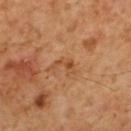Q: Was this lesion biopsied?
A: catalogued during a skin exam; not biopsied
Q: Patient demographics?
A: male, aged 58 to 62
Q: What is the anatomic site?
A: the mid back
Q: What kind of image is this?
A: 15 mm crop, total-body photography